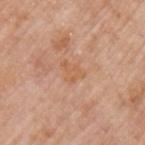notes: total-body-photography surveillance lesion; no biopsy
acquisition: total-body-photography crop, ~15 mm field of view
body site: the left upper arm
subject: male, aged around 75